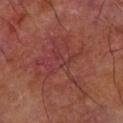The lesion was photographed on a routine skin check and not biopsied; there is no pathology result.
The tile uses cross-polarized illumination.
A male patient approximately 70 years of age.
The lesion is located on the left forearm.
This image is a 15 mm lesion crop taken from a total-body photograph.